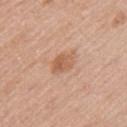Part of a total-body skin-imaging series; this lesion was reviewed on a skin check and was not flagged for biopsy. From the left upper arm. Imaged with white-light lighting. A roughly 15 mm field-of-view crop from a total-body skin photograph. The lesion-visualizer software estimated an eccentricity of roughly 0.65. It also reported an average lesion color of about L≈59 a*≈22 b*≈33 (CIELAB), a lesion–skin lightness drop of about 9, and a lesion-to-skin contrast of about 6.5 (normalized; higher = more distinct). The analysis additionally found border irregularity of about 2.5 on a 0–10 scale, a color-variation rating of about 4/10, and a peripheral color-asymmetry measure near 1.5. The subject is a female aged 48–52.